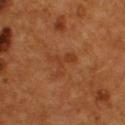| key | value |
|---|---|
| notes | imaged on a skin check; not biopsied |
| subject | male, about 55 years old |
| illumination | cross-polarized illumination |
| imaging modality | ~15 mm crop, total-body skin-cancer survey |
| body site | the upper back |
| lesion diameter | ~3.5 mm (longest diameter) |